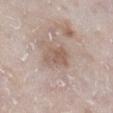This lesion was catalogued during total-body skin photography and was not selected for biopsy. A close-up tile cropped from a whole-body skin photograph, about 15 mm across. Imaged with white-light lighting. The patient is a male roughly 80 years of age. On the right lower leg.